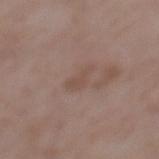| field | value |
|---|---|
| biopsy status | imaged on a skin check; not biopsied |
| acquisition | ~15 mm crop, total-body skin-cancer survey |
| patient | female, aged around 70 |
| site | the back |
| lesion size | ≈3 mm |
| automated metrics | an area of roughly 4 mm² and a shape eccentricity near 0.8; a nevus-likeness score of about 0/100 and a lesion-detection confidence of about 100/100 |
| illumination | white-light |